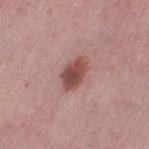The subject is a female in their mid-50s. The lesion is located on the left thigh. Automated tile analysis of the lesion measured a classifier nevus-likeness of about 95/100 and a detector confidence of about 100 out of 100 that the crop contains a lesion. The tile uses white-light illumination. About 4 mm across. A 15 mm close-up tile from a total-body photography series done for melanoma screening.